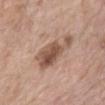{"biopsy_status": "not biopsied; imaged during a skin examination", "lighting": "white-light", "patient": {"sex": "female", "age_approx": 75}, "site": "chest", "image": {"source": "total-body photography crop", "field_of_view_mm": 15}, "lesion_size": {"long_diameter_mm_approx": 6.0}}